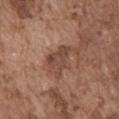Q: Is there a histopathology result?
A: no biopsy performed (imaged during a skin exam)
Q: Lesion location?
A: the abdomen
Q: Patient demographics?
A: male, in their mid- to late 70s
Q: What is the imaging modality?
A: total-body-photography crop, ~15 mm field of view